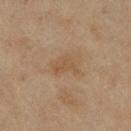{"automated_metrics": {"area_mm2_approx": 4.5, "eccentricity": 0.75, "shape_asymmetry": 0.55, "nevus_likeness_0_100": 0}, "image": {"source": "total-body photography crop", "field_of_view_mm": 15}, "patient": {"sex": "female", "age_approx": 70}, "site": "right lower leg", "lesion_size": {"long_diameter_mm_approx": 3.0}, "lighting": "cross-polarized"}